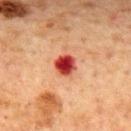Assessment:
Recorded during total-body skin imaging; not selected for excision or biopsy.
Clinical summary:
A male subject, aged 63–67. A region of skin cropped from a whole-body photographic capture, roughly 15 mm wide. The lesion is on the back. Longest diameter approximately 2.5 mm. The tile uses cross-polarized illumination.